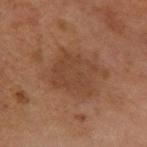Imaged during a routine full-body skin examination; the lesion was not biopsied and no histopathology is available.
A close-up tile cropped from a whole-body skin photograph, about 15 mm across.
The patient is a female about 60 years old.
From the left forearm.